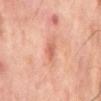{
  "biopsy_status": "not biopsied; imaged during a skin examination",
  "lesion_size": {
    "long_diameter_mm_approx": 2.5
  },
  "patient": {
    "sex": "male",
    "age_approx": 65
  },
  "image": {
    "source": "total-body photography crop",
    "field_of_view_mm": 15
  },
  "site": "mid back",
  "lighting": "cross-polarized",
  "automated_metrics": {
    "border_irregularity_0_10": 2.5,
    "color_variation_0_10": 1.0,
    "peripheral_color_asymmetry": 0.5
  }
}